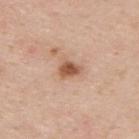{"biopsy_status": "not biopsied; imaged during a skin examination", "site": "upper back", "image": {"source": "total-body photography crop", "field_of_view_mm": 15}, "automated_metrics": {"area_mm2_approx": 4.5, "shape_asymmetry": 0.35, "cielab_L": 56, "cielab_a": 22, "cielab_b": 32, "vs_skin_darker_L": 14.0, "vs_skin_contrast_norm": 9.0, "nevus_likeness_0_100": 95}, "patient": {"sex": "male", "age_approx": 45}, "lesion_size": {"long_diameter_mm_approx": 3.0}}